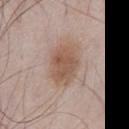{"biopsy_status": "not biopsied; imaged during a skin examination", "patient": {"sex": "male", "age_approx": 55}, "image": {"source": "total-body photography crop", "field_of_view_mm": 15}, "site": "front of the torso", "lighting": "white-light", "automated_metrics": {"border_irregularity_0_10": 1.5, "color_variation_0_10": 3.5, "peripheral_color_asymmetry": 1.0, "nevus_likeness_0_100": 80, "lesion_detection_confidence_0_100": 100}, "lesion_size": {"long_diameter_mm_approx": 5.0}}